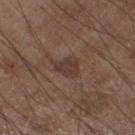Assessment:
The lesion was photographed on a routine skin check and not biopsied; there is no pathology result.
Image and clinical context:
Located on the leg. The recorded lesion diameter is about 2.5 mm. Imaged with white-light lighting. A male patient, in their mid-50s. Cropped from a total-body skin-imaging series; the visible field is about 15 mm.